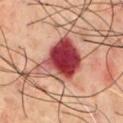Imaged during a routine full-body skin examination; the lesion was not biopsied and no histopathology is available. A male patient, aged 68–72. Longest diameter approximately 6.5 mm. The lesion is located on the front of the torso. A 15 mm close-up extracted from a 3D total-body photography capture. This is a cross-polarized tile.